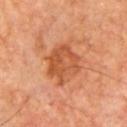follow-up: total-body-photography surveillance lesion; no biopsy | image-analysis metrics: roughly 9 lightness units darker than nearby skin and a lesion-to-skin contrast of about 7 (normalized; higher = more distinct); a classifier nevus-likeness of about 10/100 and a detector confidence of about 100 out of 100 that the crop contains a lesion | acquisition: ~15 mm tile from a whole-body skin photo | illumination: cross-polarized | size: about 4 mm | location: the front of the torso | patient: male, aged around 65.